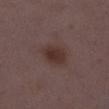Recorded during total-body skin imaging; not selected for excision or biopsy.
This is a white-light tile.
A roughly 15 mm field-of-view crop from a total-body skin photograph.
The lesion-visualizer software estimated a lesion color around L≈33 a*≈16 b*≈19 in CIELAB, roughly 7 lightness units darker than nearby skin, and a normalized border contrast of about 8. The software also gave a border-irregularity rating of about 2/10, a within-lesion color-variation index near 3/10, and peripheral color asymmetry of about 1. The analysis additionally found a nevus-likeness score of about 95/100 and lesion-presence confidence of about 100/100.
A female patient aged approximately 30.
Measured at roughly 4 mm in maximum diameter.
On the right lower leg.